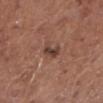workup: imaged on a skin check; not biopsied
tile lighting: white-light
site: the left lower leg
size: ≈2.5 mm
imaging modality: ~15 mm crop, total-body skin-cancer survey
patient: male, aged around 75
automated lesion analysis: an area of roughly 3.5 mm² and two-axis asymmetry of about 0.3; an average lesion color of about L≈40 a*≈19 b*≈24 (CIELAB), roughly 11 lightness units darker than nearby skin, and a normalized border contrast of about 9; a color-variation rating of about 1.5/10 and peripheral color asymmetry of about 0; a nevus-likeness score of about 50/100 and a lesion-detection confidence of about 100/100